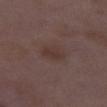follow-up = catalogued during a skin exam; not biopsied
size = about 3 mm
image source = 15 mm crop, total-body photography
patient = female, aged around 30
lighting = white-light
site = the leg